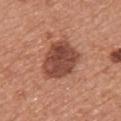Assessment:
No biopsy was performed on this lesion — it was imaged during a full skin examination and was not determined to be concerning.
Clinical summary:
This image is a 15 mm lesion crop taken from a total-body photograph. A female patient in their mid- to late 30s. On the upper back.